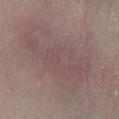Assessment:
The lesion was tiled from a total-body skin photograph and was not biopsied.
Image and clinical context:
A female patient aged approximately 55. Longest diameter approximately 10 mm. From the left lower leg. An algorithmic analysis of the crop reported a footprint of about 38 mm². The software also gave border irregularity of about 8 on a 0–10 scale, a color-variation rating of about 2.5/10, and radial color variation of about 1. And it measured a classifier nevus-likeness of about 0/100 and a detector confidence of about 55 out of 100 that the crop contains a lesion. The tile uses white-light illumination. A region of skin cropped from a whole-body photographic capture, roughly 15 mm wide.